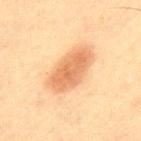The lesion was tiled from a total-body skin photograph and was not biopsied.